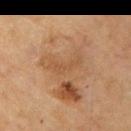Recorded during total-body skin imaging; not selected for excision or biopsy. Imaged with cross-polarized lighting. A lesion tile, about 15 mm wide, cut from a 3D total-body photograph. Automated image analysis of the tile measured an outline eccentricity of about 0.95 (0 = round, 1 = elongated) and a symmetry-axis asymmetry near 0.5. The subject is a male about 75 years old. The lesion is on the upper back.